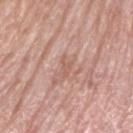Clinical impression: The lesion was tiled from a total-body skin photograph and was not biopsied. Context: A female subject, aged around 60. The tile uses white-light illumination. On the right upper arm. A close-up tile cropped from a whole-body skin photograph, about 15 mm across. Measured at roughly 3 mm in maximum diameter.